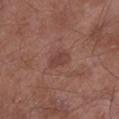<case>
  <biopsy_status>not biopsied; imaged during a skin examination</biopsy_status>
  <patient>
    <sex>male</sex>
    <age_approx>55</age_approx>
  </patient>
  <site>left lower leg</site>
  <lesion_size>
    <long_diameter_mm_approx>3.0</long_diameter_mm_approx>
  </lesion_size>
  <image>
    <source>total-body photography crop</source>
    <field_of_view_mm>15</field_of_view_mm>
  </image>
</case>